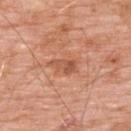Impression: The lesion was tiled from a total-body skin photograph and was not biopsied. Clinical summary: Captured under white-light illumination. A close-up tile cropped from a whole-body skin photograph, about 15 mm across. Automated tile analysis of the lesion measured a nevus-likeness score of about 0/100 and lesion-presence confidence of about 100/100. The lesion is on the upper back. A male subject in their 60s.